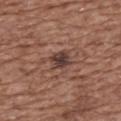  biopsy_status: not biopsied; imaged during a skin examination
  lighting: white-light
  image:
    source: total-body photography crop
    field_of_view_mm: 15
  site: back
  patient:
    sex: female
    age_approx: 75